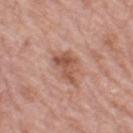Recorded during total-body skin imaging; not selected for excision or biopsy. Located on the leg. Cropped from a total-body skin-imaging series; the visible field is about 15 mm. The recorded lesion diameter is about 4.5 mm. The total-body-photography lesion software estimated a footprint of about 8.5 mm², an eccentricity of roughly 0.75, and a symmetry-axis asymmetry near 0.4. It also reported a lesion-detection confidence of about 100/100. A female subject, aged 68–72. Captured under white-light illumination.